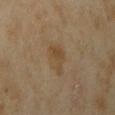This lesion was catalogued during total-body skin photography and was not selected for biopsy.
The recorded lesion diameter is about 3.5 mm.
Automated tile analysis of the lesion measured a lesion area of about 4.5 mm² and two-axis asymmetry of about 0.55. The analysis additionally found an automated nevus-likeness rating near 20 out of 100 and lesion-presence confidence of about 100/100.
The lesion is located on the right upper arm.
This is a cross-polarized tile.
A 15 mm close-up extracted from a 3D total-body photography capture.
The subject is a female in their mid-30s.